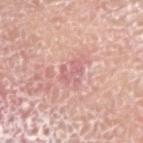Recorded during total-body skin imaging; not selected for excision or biopsy. The tile uses white-light illumination. Automated tile analysis of the lesion measured an area of roughly 3.5 mm² and an outline eccentricity of about 0.75 (0 = round, 1 = elongated). And it measured a lesion color around L≈63 a*≈27 b*≈22 in CIELAB and about 8 CIELAB-L* units darker than the surrounding skin. It also reported an automated nevus-likeness rating near 0 out of 100 and lesion-presence confidence of about 95/100. Located on the arm. A 15 mm crop from a total-body photograph taken for skin-cancer surveillance. A male patient, aged around 75.